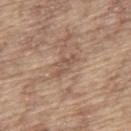workup — no biopsy performed (imaged during a skin exam) | body site — the back | size — about 3.5 mm | automated lesion analysis — a lesion area of about 4 mm² and a shape-asymmetry score of about 0.45 (0 = symmetric); a nevus-likeness score of about 0/100 and a lesion-detection confidence of about 50/100 | image — total-body-photography crop, ~15 mm field of view | patient — male, aged around 65.